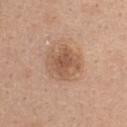{"biopsy_status": "not biopsied; imaged during a skin examination", "patient": {"sex": "female", "age_approx": 40}, "lighting": "white-light", "image": {"source": "total-body photography crop", "field_of_view_mm": 15}, "site": "upper back", "lesion_size": {"long_diameter_mm_approx": 4.5}, "automated_metrics": {"nevus_likeness_0_100": 25, "lesion_detection_confidence_0_100": 100}}